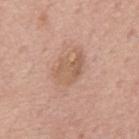Captured during whole-body skin photography for melanoma surveillance; the lesion was not biopsied.